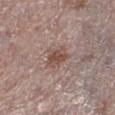The lesion was tiled from a total-body skin photograph and was not biopsied. The subject is a female about 65 years old. The lesion's longest dimension is about 3 mm. A lesion tile, about 15 mm wide, cut from a 3D total-body photograph. The tile uses white-light illumination. On the leg.